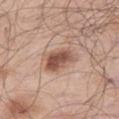biopsy status: total-body-photography surveillance lesion; no biopsy
imaging modality: total-body-photography crop, ~15 mm field of view
subject: male, aged around 70
size: about 4 mm
TBP lesion metrics: a footprint of about 9 mm², an outline eccentricity of about 0.75 (0 = round, 1 = elongated), and a symmetry-axis asymmetry near 0.2; an average lesion color of about L≈53 a*≈21 b*≈28 (CIELAB) and about 14 CIELAB-L* units darker than the surrounding skin; a border-irregularity rating of about 2/10 and radial color variation of about 2; a nevus-likeness score of about 85/100 and a lesion-detection confidence of about 100/100
anatomic site: the leg